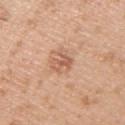{
  "patient": {
    "sex": "male",
    "age_approx": 30
  },
  "image": {
    "source": "total-body photography crop",
    "field_of_view_mm": 15
  },
  "lighting": "white-light",
  "automated_metrics": {
    "cielab_L": 60,
    "cielab_a": 22,
    "cielab_b": 32,
    "vs_skin_darker_L": 10.0,
    "vs_skin_contrast_norm": 6.0,
    "color_variation_0_10": 4.5,
    "peripheral_color_asymmetry": 2.0,
    "lesion_detection_confidence_0_100": 100
  },
  "site": "right upper arm"
}